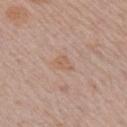| feature | finding |
|---|---|
| follow-up | imaged on a skin check; not biopsied |
| TBP lesion metrics | a lesion–skin lightness drop of about 5 and a lesion-to-skin contrast of about 5 (normalized; higher = more distinct); an automated nevus-likeness rating near 0 out of 100 and lesion-presence confidence of about 100/100 |
| size | ≈2.5 mm |
| image | ~15 mm crop, total-body skin-cancer survey |
| lighting | white-light |
| patient | male, aged 68 to 72 |
| body site | the right upper arm |